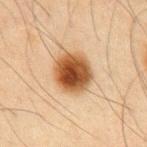biopsy status: catalogued during a skin exam; not biopsied
lighting: cross-polarized
subject: male, approximately 65 years of age
site: the chest
image: 15 mm crop, total-body photography